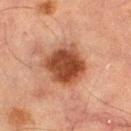Clinical impression: Part of a total-body skin-imaging series; this lesion was reviewed on a skin check and was not flagged for biopsy. Clinical summary: Measured at roughly 5 mm in maximum diameter. The tile uses cross-polarized illumination. The patient is a male aged 63–67. On the left thigh. A 15 mm crop from a total-body photograph taken for skin-cancer surveillance.